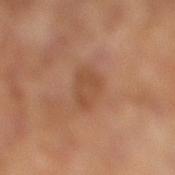Q: Was a biopsy performed?
A: catalogued during a skin exam; not biopsied
Q: How was this image acquired?
A: total-body-photography crop, ~15 mm field of view
Q: Lesion location?
A: the leg
Q: What are the patient's age and sex?
A: female, aged approximately 60